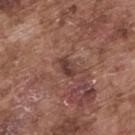<lesion>
  <biopsy_status>not biopsied; imaged during a skin examination</biopsy_status>
  <patient>
    <sex>male</sex>
    <age_approx>75</age_approx>
  </patient>
  <image>
    <source>total-body photography crop</source>
    <field_of_view_mm>15</field_of_view_mm>
  </image>
  <automated_metrics>
    <cielab_L>39</cielab_L>
    <cielab_a>20</cielab_a>
    <cielab_b>23</cielab_b>
    <vs_skin_darker_L>9.0</vs_skin_darker_L>
    <vs_skin_contrast_norm>8.0</vs_skin_contrast_norm>
    <nevus_likeness_0_100>0</nevus_likeness_0_100>
    <lesion_detection_confidence_0_100>100</lesion_detection_confidence_0_100>
  </automated_metrics>
  <site>back</site>
  <lesion_size>
    <long_diameter_mm_approx>2.5</long_diameter_mm_approx>
  </lesion_size>
</lesion>